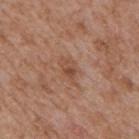biopsy status: no biopsy performed (imaged during a skin exam)
subject: male, in their mid-60s
automated lesion analysis: a lesion area of about 5 mm², an outline eccentricity of about 0.8 (0 = round, 1 = elongated), and a symmetry-axis asymmetry near 0.25; a border-irregularity rating of about 2.5/10, internal color variation of about 4.5 on a 0–10 scale, and peripheral color asymmetry of about 1.5; an automated nevus-likeness rating near 0 out of 100 and a detector confidence of about 100 out of 100 that the crop contains a lesion
imaging modality: total-body-photography crop, ~15 mm field of view
tile lighting: white-light
location: the back